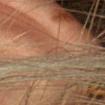Assessment:
Part of a total-body skin-imaging series; this lesion was reviewed on a skin check and was not flagged for biopsy.
Context:
A female subject aged around 25. A close-up tile cropped from a whole-body skin photograph, about 15 mm across. Located on the head or neck.